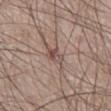follow-up: imaged on a skin check; not biopsied | imaging modality: 15 mm crop, total-body photography | patient: male, about 60 years old | body site: the right lower leg.